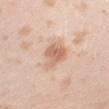Assessment: The lesion was tiled from a total-body skin photograph and was not biopsied. Clinical summary: A female subject, about 25 years old. A 15 mm close-up tile from a total-body photography series done for melanoma screening. Approximately 4 mm at its widest. On the right upper arm. Imaged with white-light lighting.